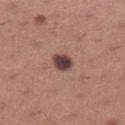Captured during whole-body skin photography for melanoma surveillance; the lesion was not biopsied. A 15 mm close-up extracted from a 3D total-body photography capture. Longest diameter approximately 2.5 mm. A female subject aged approximately 30. The lesion is located on the left lower leg. Automated image analysis of the tile measured a lesion area of about 5 mm², an eccentricity of roughly 0.5, and a symmetry-axis asymmetry near 0.2. It also reported an average lesion color of about L≈42 a*≈19 b*≈20 (CIELAB), about 16 CIELAB-L* units darker than the surrounding skin, and a normalized lesion–skin contrast near 12. It also reported a classifier nevus-likeness of about 50/100 and a detector confidence of about 100 out of 100 that the crop contains a lesion.